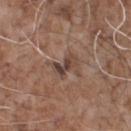This lesion was catalogued during total-body skin photography and was not selected for biopsy.
This is a white-light tile.
A roughly 15 mm field-of-view crop from a total-body skin photograph.
From the chest.
Automated image analysis of the tile measured a lesion area of about 4 mm² and an eccentricity of roughly 0.85. The software also gave a mean CIELAB color near L≈41 a*≈17 b*≈22, roughly 10 lightness units darker than nearby skin, and a normalized lesion–skin contrast near 8.5. It also reported border irregularity of about 4.5 on a 0–10 scale, internal color variation of about 2 on a 0–10 scale, and radial color variation of about 0. And it measured a nevus-likeness score of about 0/100.
About 3 mm across.
The subject is a male in their 70s.